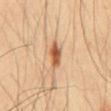workup = imaged on a skin check; not biopsied
lesion diameter = about 3 mm
TBP lesion metrics = an average lesion color of about L≈58 a*≈24 b*≈38 (CIELAB), roughly 15 lightness units darker than nearby skin, and a normalized border contrast of about 9.5; a border-irregularity index near 2.5/10 and a color-variation rating of about 5.5/10; a nevus-likeness score of about 95/100
subject = male, aged around 50
anatomic site = the mid back
image source = ~15 mm crop, total-body skin-cancer survey
tile lighting = cross-polarized illumination